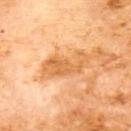This lesion was catalogued during total-body skin photography and was not selected for biopsy. Cropped from a whole-body photographic skin survey; the tile spans about 15 mm. From the upper back. The recorded lesion diameter is about 6 mm. A male subject, approximately 70 years of age.